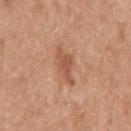{"biopsy_status": "not biopsied; imaged during a skin examination", "site": "right upper arm", "image": {"source": "total-body photography crop", "field_of_view_mm": 15}, "patient": {"sex": "female", "age_approx": 50}}